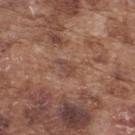Q: Is there a histopathology result?
A: total-body-photography surveillance lesion; no biopsy
Q: Illumination type?
A: white-light illumination
Q: Who is the patient?
A: male, roughly 75 years of age
Q: What did automated image analysis measure?
A: a lesion area of about 3 mm², a shape eccentricity near 0.85, and a shape-asymmetry score of about 0.25 (0 = symmetric); a border-irregularity index near 2.5/10, a color-variation rating of about 2/10, and radial color variation of about 0.5
Q: What is the anatomic site?
A: the upper back
Q: What kind of image is this?
A: ~15 mm tile from a whole-body skin photo